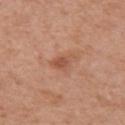follow-up=imaged on a skin check; not biopsied
size=≈2.5 mm
image source=15 mm crop, total-body photography
subject=female, aged approximately 50
location=the left upper arm
automated lesion analysis=an area of roughly 4 mm², a shape eccentricity near 0.6, and two-axis asymmetry of about 0.35